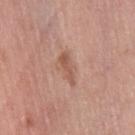Context:
Longest diameter approximately 4 mm. On the right thigh. A female subject, aged around 65. Automated tile analysis of the lesion measured an eccentricity of roughly 0.95. The software also gave a lesion color around L≈55 a*≈22 b*≈29 in CIELAB. The software also gave border irregularity of about 5 on a 0–10 scale and a within-lesion color-variation index near 1/10. And it measured a classifier nevus-likeness of about 0/100. A lesion tile, about 15 mm wide, cut from a 3D total-body photograph. The tile uses white-light illumination.